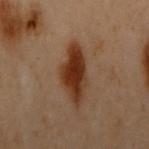follow-up — no biopsy performed (imaged during a skin exam)
illumination — cross-polarized
size — ≈6.5 mm
subject — female, approximately 60 years of age
image source — ~15 mm crop, total-body skin-cancer survey
site — the upper back